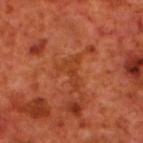Assessment: No biopsy was performed on this lesion — it was imaged during a full skin examination and was not determined to be concerning. Acquisition and patient details: A male subject, about 70 years old. From the back. A 15 mm close-up tile from a total-body photography series done for melanoma screening. The recorded lesion diameter is about 4 mm. Imaged with cross-polarized lighting.